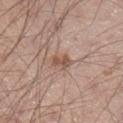Clinical summary: A male patient, aged around 35. Automated image analysis of the tile measured an area of roughly 3 mm², an outline eccentricity of about 0.8 (0 = round, 1 = elongated), and a shape-asymmetry score of about 0.35 (0 = symmetric). The analysis additionally found an average lesion color of about L≈52 a*≈19 b*≈27 (CIELAB), roughly 10 lightness units darker than nearby skin, and a normalized lesion–skin contrast near 7.5. And it measured a border-irregularity index near 3/10, a within-lesion color-variation index near 1/10, and a peripheral color-asymmetry measure near 0.5. This image is a 15 mm lesion crop taken from a total-body photograph. The lesion is on the left lower leg.